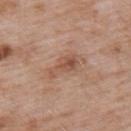The lesion was tiled from a total-body skin photograph and was not biopsied. From the upper back. The lesion-visualizer software estimated about 9 CIELAB-L* units darker than the surrounding skin and a normalized border contrast of about 6.5. And it measured a border-irregularity index near 5/10, internal color variation of about 4 on a 0–10 scale, and peripheral color asymmetry of about 1.5. A 15 mm close-up tile from a total-body photography series done for melanoma screening. About 4 mm across. A male patient in their mid-50s. Captured under white-light illumination.